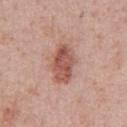Recorded during total-body skin imaging; not selected for excision or biopsy.
On the chest.
A roughly 15 mm field-of-view crop from a total-body skin photograph.
A male patient aged around 40.
Captured under white-light illumination.
Measured at roughly 5 mm in maximum diameter.